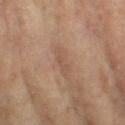<tbp_lesion>
<biopsy_status>not biopsied; imaged during a skin examination</biopsy_status>
<lighting>cross-polarized</lighting>
<site>left thigh</site>
<image>
  <source>total-body photography crop</source>
  <field_of_view_mm>15</field_of_view_mm>
</image>
<automated_metrics>
  <area_mm2_approx>3.0</area_mm2_approx>
  <cielab_L>52</cielab_L>
  <cielab_a>18</cielab_a>
  <cielab_b>28</cielab_b>
  <vs_skin_darker_L>7.0</vs_skin_darker_L>
  <border_irregularity_0_10>5.5</border_irregularity_0_10>
  <color_variation_0_10>0.0</color_variation_0_10>
  <peripheral_color_asymmetry>0.0</peripheral_color_asymmetry>
  <nevus_likeness_0_100>0</nevus_likeness_0_100>
  <lesion_detection_confidence_0_100>60</lesion_detection_confidence_0_100>
</automated_metrics>
<patient>
  <sex>female</sex>
  <age_approx>75</age_approx>
</patient>
</tbp_lesion>